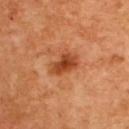Findings:
- workup — no biopsy performed (imaged during a skin exam)
- size — ~3.5 mm (longest diameter)
- tile lighting — cross-polarized
- acquisition — total-body-photography crop, ~15 mm field of view
- subject — male, aged around 60
- TBP lesion metrics — an average lesion color of about L≈45 a*≈30 b*≈39 (CIELAB), a lesion–skin lightness drop of about 12, and a normalized lesion–skin contrast near 9; border irregularity of about 3 on a 0–10 scale; a nevus-likeness score of about 80/100 and a detector confidence of about 100 out of 100 that the crop contains a lesion
- location — the upper back